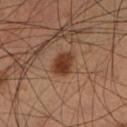workup=no biopsy performed (imaged during a skin exam); lesion size=about 3 mm; imaging modality=15 mm crop, total-body photography; site=the left lower leg; subject=male, aged 58 to 62; illumination=cross-polarized.